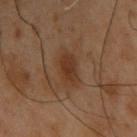Clinical impression: The lesion was tiled from a total-body skin photograph and was not biopsied. Image and clinical context: An algorithmic analysis of the crop reported a footprint of about 6 mm², a shape eccentricity near 0.75, and two-axis asymmetry of about 0.2. The analysis additionally found a lesion color around L≈27 a*≈16 b*≈25 in CIELAB and about 6 CIELAB-L* units darker than the surrounding skin. The analysis additionally found lesion-presence confidence of about 100/100. The lesion is located on the chest. A male patient, aged 53 to 57. This image is a 15 mm lesion crop taken from a total-body photograph.